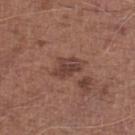| feature | finding |
|---|---|
| follow-up | no biopsy performed (imaged during a skin exam) |
| site | the leg |
| subject | male, roughly 75 years of age |
| image source | 15 mm crop, total-body photography |
| tile lighting | white-light |
| image-analysis metrics | two-axis asymmetry of about 0.3; an average lesion color of about L≈41 a*≈20 b*≈24 (CIELAB), roughly 9 lightness units darker than nearby skin, and a normalized lesion–skin contrast near 7.5; a nevus-likeness score of about 5/100 and a lesion-detection confidence of about 100/100 |